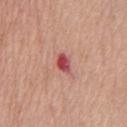workup: no biopsy performed (imaged during a skin exam); lesion diameter: ≈2.5 mm; site: the chest; patient: female, in their mid-60s; image source: 15 mm crop, total-body photography.